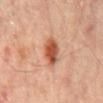Q: Was a biopsy performed?
A: imaged on a skin check; not biopsied
Q: What is the lesion's diameter?
A: ≈3.5 mm
Q: What kind of image is this?
A: ~15 mm tile from a whole-body skin photo
Q: Automated lesion metrics?
A: a lesion color around L≈53 a*≈26 b*≈34 in CIELAB, a lesion–skin lightness drop of about 14, and a normalized border contrast of about 9.5; a nevus-likeness score of about 95/100 and a detector confidence of about 100 out of 100 that the crop contains a lesion
Q: What is the anatomic site?
A: the mid back
Q: What lighting was used for the tile?
A: cross-polarized
Q: Patient demographics?
A: male, roughly 65 years of age